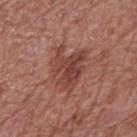Q: Is there a histopathology result?
A: no biopsy performed (imaged during a skin exam)
Q: Who is the patient?
A: male, aged around 70
Q: How was the tile lit?
A: white-light illumination
Q: Automated lesion metrics?
A: a lesion color around L≈43 a*≈24 b*≈26 in CIELAB, about 9 CIELAB-L* units darker than the surrounding skin, and a normalized border contrast of about 7.5; border irregularity of about 5.5 on a 0–10 scale and peripheral color asymmetry of about 2
Q: Where on the body is the lesion?
A: the right upper arm
Q: What kind of image is this?
A: 15 mm crop, total-body photography